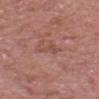Impression: No biopsy was performed on this lesion — it was imaged during a full skin examination and was not determined to be concerning. Context: The tile uses white-light illumination. The patient is a male aged 63–67. This image is a 15 mm lesion crop taken from a total-body photograph. From the head or neck.